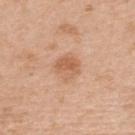Findings:
* workup: no biopsy performed (imaged during a skin exam)
* lesion diameter: ~3 mm (longest diameter)
* imaging modality: ~15 mm tile from a whole-body skin photo
* body site: the upper back
* subject: male, roughly 70 years of age
* TBP lesion metrics: a footprint of about 7 mm² and two-axis asymmetry of about 0.2; a mean CIELAB color near L≈61 a*≈22 b*≈35, roughly 9 lightness units darker than nearby skin, and a normalized lesion–skin contrast near 6